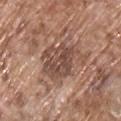Q: Was this lesion biopsied?
A: no biopsy performed (imaged during a skin exam)
Q: Lesion location?
A: the chest
Q: What are the patient's age and sex?
A: male, aged 68–72
Q: What is the imaging modality?
A: ~15 mm crop, total-body skin-cancer survey